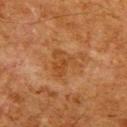Recorded during total-body skin imaging; not selected for excision or biopsy.